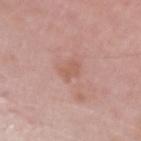Q: Was a biopsy performed?
A: no biopsy performed (imaged during a skin exam)
Q: What is the anatomic site?
A: the left upper arm
Q: How was the tile lit?
A: white-light illumination
Q: What did automated image analysis measure?
A: a border-irregularity index near 4/10, a color-variation rating of about 1/10, and radial color variation of about 0.5; a detector confidence of about 100 out of 100 that the crop contains a lesion
Q: How large is the lesion?
A: about 2.5 mm
Q: What kind of image is this?
A: total-body-photography crop, ~15 mm field of view
Q: Patient demographics?
A: female, aged 43 to 47